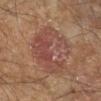| field | value |
|---|---|
| workup | no biopsy performed (imaged during a skin exam) |